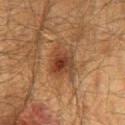Recorded during total-body skin imaging; not selected for excision or biopsy. An algorithmic analysis of the crop reported a footprint of about 10 mm², a shape eccentricity near 0.55, and a shape-asymmetry score of about 0.35 (0 = symmetric). It also reported a lesion color around L≈32 a*≈18 b*≈26 in CIELAB, a lesion–skin lightness drop of about 8, and a normalized lesion–skin contrast near 8. The analysis additionally found a border-irregularity index near 3.5/10, internal color variation of about 5.5 on a 0–10 scale, and a peripheral color-asymmetry measure near 1.5. The lesion is on the mid back. The tile uses cross-polarized illumination. The patient is a male in their 60s. A 15 mm crop from a total-body photograph taken for skin-cancer surveillance. About 4 mm across.